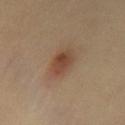image — ~15 mm crop, total-body skin-cancer survey; diameter — about 4.5 mm; patient — female, aged 38 to 42; anatomic site — the front of the torso; lighting — cross-polarized.